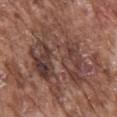Assessment:
Recorded during total-body skin imaging; not selected for excision or biopsy.
Clinical summary:
The subject is a male aged 73 to 77. A 15 mm close-up tile from a total-body photography series done for melanoma screening. Measured at roughly 9 mm in maximum diameter. The lesion is located on the back.